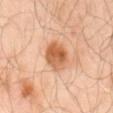Recorded during total-body skin imaging; not selected for excision or biopsy. The subject is a male about 65 years old. On the mid back. This image is a 15 mm lesion crop taken from a total-body photograph.